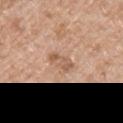* workup · catalogued during a skin exam; not biopsied
* tile lighting · white-light
* subject · male, aged 48–52
* imaging modality · total-body-photography crop, ~15 mm field of view
* location · the arm